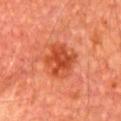{"biopsy_status": "not biopsied; imaged during a skin examination", "image": {"source": "total-body photography crop", "field_of_view_mm": 15}, "automated_metrics": {"area_mm2_approx": 13.0, "eccentricity": 0.55, "shape_asymmetry": 0.2, "cielab_L": 46, "cielab_a": 34, "cielab_b": 38, "vs_skin_darker_L": 10.0}, "site": "chest", "lighting": "cross-polarized", "patient": {"sex": "male", "age_approx": 65}}